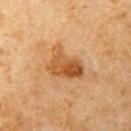Findings:
– illumination · cross-polarized illumination
– TBP lesion metrics · a lesion color around L≈43 a*≈20 b*≈34 in CIELAB
– lesion diameter · ~5 mm (longest diameter)
– imaging modality · 15 mm crop, total-body photography
– body site · the left upper arm
– subject · male, roughly 65 years of age